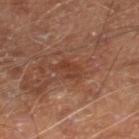| key | value |
|---|---|
| image | 15 mm crop, total-body photography |
| subject | male, aged approximately 70 |
| anatomic site | the right lower leg |